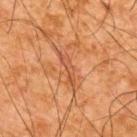  image:
    source: total-body photography crop
    field_of_view_mm: 15
  patient:
    sex: male
    age_approx: 65
  lesion_size:
    long_diameter_mm_approx: 4.0
  site: upper back
  automated_metrics:
    area_mm2_approx: 4.0
    eccentricity: 0.95
    shape_asymmetry: 0.5
    cielab_L: 43
    cielab_a: 23
    cielab_b: 32
    vs_skin_darker_L: 7.0
    vs_skin_contrast_norm: 5.5
    border_irregularity_0_10: 6.5
    color_variation_0_10: 0.0
    peripheral_color_asymmetry: 0.0